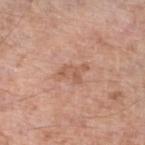| feature | finding |
|---|---|
| workup | total-body-photography surveillance lesion; no biopsy |
| body site | the leg |
| acquisition | total-body-photography crop, ~15 mm field of view |
| diameter | ≈3.5 mm |
| lighting | white-light |
| automated metrics | an outline eccentricity of about 0.8 (0 = round, 1 = elongated) and a symmetry-axis asymmetry near 0.45; a lesion–skin lightness drop of about 8 and a normalized lesion–skin contrast near 5.5; a border-irregularity rating of about 5/10, a color-variation rating of about 2.5/10, and a peripheral color-asymmetry measure near 1; a lesion-detection confidence of about 100/100 |
| subject | male, aged 78–82 |